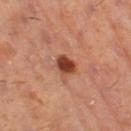Clinical impression: The lesion was photographed on a routine skin check and not biopsied; there is no pathology result. Acquisition and patient details: A female patient, aged 38 to 42. A 15 mm crop from a total-body photograph taken for skin-cancer surveillance. On the leg.